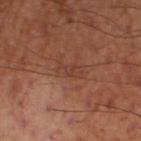Q: Was this lesion biopsied?
A: catalogued during a skin exam; not biopsied
Q: Automated lesion metrics?
A: a mean CIELAB color near L≈39 a*≈24 b*≈27, about 6 CIELAB-L* units darker than the surrounding skin, and a normalized lesion–skin contrast near 5; a border-irregularity index near 5.5/10 and a color-variation rating of about 0/10
Q: Lesion size?
A: ~2.5 mm (longest diameter)
Q: Patient demographics?
A: male, in their mid-50s
Q: What is the imaging modality?
A: total-body-photography crop, ~15 mm field of view
Q: Where on the body is the lesion?
A: the right thigh
Q: Illumination type?
A: cross-polarized illumination